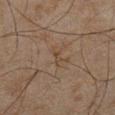subject=male, aged approximately 45
illumination=cross-polarized
size=≈3 mm
anatomic site=the left lower leg
acquisition=total-body-photography crop, ~15 mm field of view
automated lesion analysis=a lesion area of about 2.5 mm², a shape eccentricity near 0.95, and two-axis asymmetry of about 0.5; a nevus-likeness score of about 0/100 and lesion-presence confidence of about 95/100